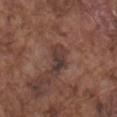Captured during whole-body skin photography for melanoma surveillance; the lesion was not biopsied.
Longest diameter approximately 3.5 mm.
Imaged with white-light lighting.
A male subject in their mid- to late 70s.
The lesion is located on the mid back.
A region of skin cropped from a whole-body photographic capture, roughly 15 mm wide.
The lesion-visualizer software estimated a symmetry-axis asymmetry near 0.3. It also reported border irregularity of about 3.5 on a 0–10 scale, a color-variation rating of about 5/10, and radial color variation of about 1.5. It also reported a nevus-likeness score of about 0/100.